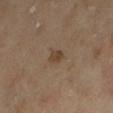<tbp_lesion>
  <biopsy_status>not biopsied; imaged during a skin examination</biopsy_status>
  <patient>
    <sex>male</sex>
    <age_approx>65</age_approx>
  </patient>
  <automated_metrics>
    <cielab_L>41</cielab_L>
    <cielab_a>14</cielab_a>
    <cielab_b>28</cielab_b>
    <vs_skin_darker_L>8.0</vs_skin_darker_L>
    <vs_skin_contrast_norm>7.0</vs_skin_contrast_norm>
    <border_irregularity_0_10>2.5</border_irregularity_0_10>
    <color_variation_0_10>2.0</color_variation_0_10>
    <peripheral_color_asymmetry>0.5</peripheral_color_asymmetry>
  </automated_metrics>
  <image>
    <source>total-body photography crop</source>
    <field_of_view_mm>15</field_of_view_mm>
  </image>
  <lighting>cross-polarized</lighting>
  <lesion_size>
    <long_diameter_mm_approx>2.0</long_diameter_mm_approx>
  </lesion_size>
  <site>leg</site>
</tbp_lesion>